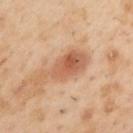workup: total-body-photography surveillance lesion; no biopsy | lighting: cross-polarized | image source: 15 mm crop, total-body photography | patient: male, aged 53 to 57 | lesion diameter: ~6.5 mm (longest diameter) | site: the left upper arm.